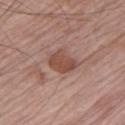Part of a total-body skin-imaging series; this lesion was reviewed on a skin check and was not flagged for biopsy. A male patient aged 63 to 67. From the left upper arm. A lesion tile, about 15 mm wide, cut from a 3D total-body photograph.